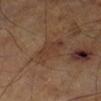notes: imaged on a skin check; not biopsied | imaging modality: ~15 mm crop, total-body skin-cancer survey | lesion size: about 4.5 mm | lighting: cross-polarized illumination | patient: male, aged 63–67 | anatomic site: the left leg | automated lesion analysis: a classifier nevus-likeness of about 0/100.